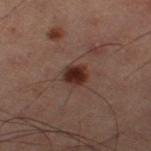Captured during whole-body skin photography for melanoma surveillance; the lesion was not biopsied.
Cropped from a whole-body photographic skin survey; the tile spans about 15 mm.
The tile uses cross-polarized illumination.
A male subject aged 58 to 62.
The recorded lesion diameter is about 2.5 mm.
The lesion is located on the leg.
Automated tile analysis of the lesion measured a lesion color around L≈20 a*≈16 b*≈18 in CIELAB, roughly 10 lightness units darker than nearby skin, and a lesion-to-skin contrast of about 12 (normalized; higher = more distinct). The analysis additionally found a border-irregularity rating of about 1.5/10, internal color variation of about 2.5 on a 0–10 scale, and radial color variation of about 1. It also reported a lesion-detection confidence of about 100/100.